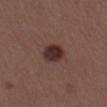Part of a total-body skin-imaging series; this lesion was reviewed on a skin check and was not flagged for biopsy.
A lesion tile, about 15 mm wide, cut from a 3D total-body photograph.
Imaged with white-light lighting.
Longest diameter approximately 3 mm.
Located on the left thigh.
A female subject aged 38–42.
An algorithmic analysis of the crop reported a symmetry-axis asymmetry near 0.15. It also reported an average lesion color of about L≈30 a*≈18 b*≈21 (CIELAB) and about 12 CIELAB-L* units darker than the surrounding skin. The analysis additionally found a detector confidence of about 100 out of 100 that the crop contains a lesion.